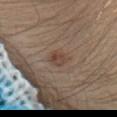Impression:
No biopsy was performed on this lesion — it was imaged during a full skin examination and was not determined to be concerning.
Acquisition and patient details:
A lesion tile, about 15 mm wide, cut from a 3D total-body photograph. From the head or neck. Imaged with white-light lighting. The recorded lesion diameter is about 2.5 mm. A female patient about 25 years old.